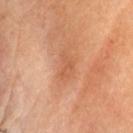Q: What are the patient's age and sex?
A: female, in their mid- to late 60s
Q: Automated lesion metrics?
A: an area of roughly 4 mm², a shape eccentricity near 0.85, and a symmetry-axis asymmetry near 0.45; an average lesion color of about L≈56 a*≈25 b*≈36 (CIELAB) and a normalized lesion–skin contrast near 5; a border-irregularity rating of about 4.5/10, a within-lesion color-variation index near 1.5/10, and radial color variation of about 0.5
Q: What kind of image is this?
A: 15 mm crop, total-body photography
Q: What is the anatomic site?
A: the head or neck
Q: Illumination type?
A: cross-polarized illumination